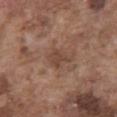The lesion was tiled from a total-body skin photograph and was not biopsied.
A male subject in their mid- to late 70s.
On the front of the torso.
A 15 mm crop from a total-body photograph taken for skin-cancer surveillance.
Automated tile analysis of the lesion measured a border-irregularity index near 3.5/10, internal color variation of about 1.5 on a 0–10 scale, and peripheral color asymmetry of about 0.5.
The tile uses white-light illumination.